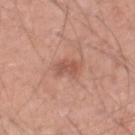Clinical impression:
Part of a total-body skin-imaging series; this lesion was reviewed on a skin check and was not flagged for biopsy.
Context:
The lesion-visualizer software estimated a lesion area of about 3.5 mm², a shape eccentricity near 0.8, and a shape-asymmetry score of about 0.3 (0 = symmetric). And it measured a lesion color around L≈54 a*≈25 b*≈28 in CIELAB, about 9 CIELAB-L* units darker than the surrounding skin, and a normalized border contrast of about 6. The analysis additionally found an automated nevus-likeness rating near 20 out of 100 and a lesion-detection confidence of about 100/100. The subject is a male aged 53–57. A 15 mm close-up extracted from a 3D total-body photography capture. Located on the right lower leg. About 2.5 mm across. The tile uses white-light illumination.